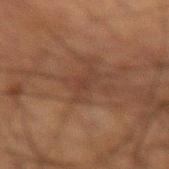Clinical impression:
The lesion was photographed on a routine skin check and not biopsied; there is no pathology result.
Image and clinical context:
A male patient, in their 60s. Located on the arm. This is a cross-polarized tile. Longest diameter approximately 4 mm. A close-up tile cropped from a whole-body skin photograph, about 15 mm across.